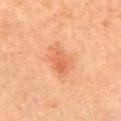Notes:
• biopsy status — imaged on a skin check; not biopsied
• site — the arm
• illumination — cross-polarized illumination
• TBP lesion metrics — a footprint of about 8.5 mm², a shape eccentricity near 0.75, and two-axis asymmetry of about 0.3; radial color variation of about 1.5; a detector confidence of about 100 out of 100 that the crop contains a lesion
• size — ~4 mm (longest diameter)
• image source — total-body-photography crop, ~15 mm field of view
• patient — female, approximately 65 years of age